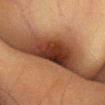The lesion was tiled from a total-body skin photograph and was not biopsied. The lesion is located on the head or neck. Captured under cross-polarized illumination. A 15 mm close-up tile from a total-body photography series done for melanoma screening. A female subject, aged approximately 40.